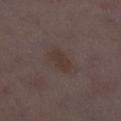Cropped from a total-body skin-imaging series; the visible field is about 15 mm. The lesion is on the leg. The patient is a female about 30 years old.